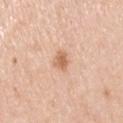Clinical impression: Imaged during a routine full-body skin examination; the lesion was not biopsied and no histopathology is available. Background: An algorithmic analysis of the crop reported border irregularity of about 2.5 on a 0–10 scale, a within-lesion color-variation index near 2/10, and a peripheral color-asymmetry measure near 1. A 15 mm close-up tile from a total-body photography series done for melanoma screening. Longest diameter approximately 3 mm. The subject is a male aged approximately 50. The lesion is located on the arm.